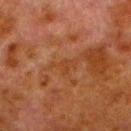Impression: No biopsy was performed on this lesion — it was imaged during a full skin examination and was not determined to be concerning. Clinical summary: The recorded lesion diameter is about 3 mm. A 15 mm crop from a total-body photograph taken for skin-cancer surveillance. An algorithmic analysis of the crop reported a lesion area of about 3.5 mm² and an eccentricity of roughly 0.8. The analysis additionally found a lesion color around L≈33 a*≈21 b*≈31 in CIELAB, about 4 CIELAB-L* units darker than the surrounding skin, and a lesion-to-skin contrast of about 5.5 (normalized; higher = more distinct). The analysis additionally found border irregularity of about 6 on a 0–10 scale, a within-lesion color-variation index near 0.5/10, and a peripheral color-asymmetry measure near 0. The subject is a male aged 78 to 82. From the left lower leg. The tile uses cross-polarized illumination.